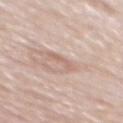Q: Is there a histopathology result?
A: catalogued during a skin exam; not biopsied
Q: Lesion location?
A: the back
Q: What kind of image is this?
A: ~15 mm crop, total-body skin-cancer survey
Q: Automated lesion metrics?
A: a border-irregularity rating of about 4.5/10, a color-variation rating of about 0/10, and a peripheral color-asymmetry measure near 0
Q: What are the patient's age and sex?
A: male, about 80 years old